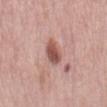Located on the mid back.
Cropped from a whole-body photographic skin survey; the tile spans about 15 mm.
The subject is a female about 65 years old.